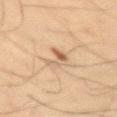This lesion was catalogued during total-body skin photography and was not selected for biopsy.
About 3.5 mm across.
A male patient, in their mid-30s.
From the right upper arm.
Imaged with cross-polarized lighting.
A roughly 15 mm field-of-view crop from a total-body skin photograph.